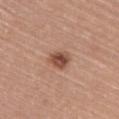Q: What did automated image analysis measure?
A: a nevus-likeness score of about 95/100
Q: Who is the patient?
A: female, aged around 65
Q: Illumination type?
A: white-light illumination
Q: What kind of image is this?
A: ~15 mm tile from a whole-body skin photo
Q: What is the lesion's diameter?
A: about 3 mm
Q: What is the anatomic site?
A: the upper back